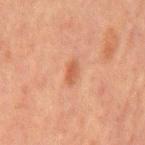Part of a total-body skin-imaging series; this lesion was reviewed on a skin check and was not flagged for biopsy.
A lesion tile, about 15 mm wide, cut from a 3D total-body photograph.
Measured at roughly 2.5 mm in maximum diameter.
Automated image analysis of the tile measured a footprint of about 3 mm², an eccentricity of roughly 0.85, and a shape-asymmetry score of about 0.35 (0 = symmetric). The analysis additionally found about 8 CIELAB-L* units darker than the surrounding skin and a lesion-to-skin contrast of about 6.5 (normalized; higher = more distinct). It also reported a border-irregularity rating of about 3/10, a color-variation rating of about 0.5/10, and peripheral color asymmetry of about 0.
Captured under cross-polarized illumination.
A male patient approximately 65 years of age.
On the abdomen.